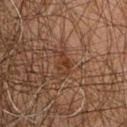Assessment: Part of a total-body skin-imaging series; this lesion was reviewed on a skin check and was not flagged for biopsy. Clinical summary: A male subject aged approximately 60. A region of skin cropped from a whole-body photographic capture, roughly 15 mm wide. Imaged with cross-polarized lighting. From the chest. The lesion's longest dimension is about 3 mm.